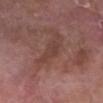No biopsy was performed on this lesion — it was imaged during a full skin examination and was not determined to be concerning. A male patient aged 78 to 82. On the leg. The tile uses white-light illumination. A lesion tile, about 15 mm wide, cut from a 3D total-body photograph. The recorded lesion diameter is about 4 mm.